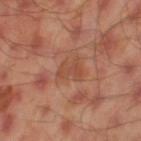The lesion was photographed on a routine skin check and not biopsied; there is no pathology result. On the left thigh. Imaged with cross-polarized lighting. A male patient, in their mid-40s. The recorded lesion diameter is about 2.5 mm. Cropped from a total-body skin-imaging series; the visible field is about 15 mm.